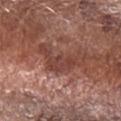<case>
  <biopsy_status>not biopsied; imaged during a skin examination</biopsy_status>
  <lesion_size>
    <long_diameter_mm_approx>6.0</long_diameter_mm_approx>
  </lesion_size>
  <image>
    <source>total-body photography crop</source>
    <field_of_view_mm>15</field_of_view_mm>
  </image>
  <site>right forearm</site>
  <patient>
    <sex>male</sex>
    <age_approx>70</age_approx>
  </patient>
  <lighting>white-light</lighting>
</case>